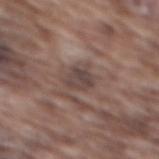Recorded during total-body skin imaging; not selected for excision or biopsy. The lesion is on the mid back. The patient is a male aged 73 to 77. Measured at roughly 3 mm in maximum diameter. A lesion tile, about 15 mm wide, cut from a 3D total-body photograph. Imaged with white-light lighting. The total-body-photography lesion software estimated an eccentricity of roughly 0.5 and two-axis asymmetry of about 0.25. The software also gave a lesion color around L≈43 a*≈15 b*≈19 in CIELAB.